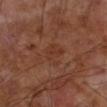Recorded during total-body skin imaging; not selected for excision or biopsy. The patient is a male approximately 70 years of age. On the left forearm. A lesion tile, about 15 mm wide, cut from a 3D total-body photograph.